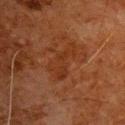* workup — no biopsy performed (imaged during a skin exam)
* acquisition — ~15 mm tile from a whole-body skin photo
* site — the chest
* illumination — cross-polarized illumination
* lesion size — about 5 mm
* subject — male, aged around 80
* automated metrics — a mean CIELAB color near L≈26 a*≈21 b*≈28, a lesion–skin lightness drop of about 5, and a normalized border contrast of about 5.5; border irregularity of about 7 on a 0–10 scale and radial color variation of about 0.5; an automated nevus-likeness rating near 0 out of 100 and a detector confidence of about 100 out of 100 that the crop contains a lesion